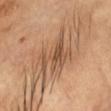The lesion was tiled from a total-body skin photograph and was not biopsied. From the head or neck. The lesion's longest dimension is about 7 mm. This is a cross-polarized tile. A 15 mm close-up extracted from a 3D total-body photography capture. The patient is a female aged around 65.